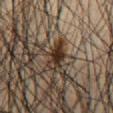<record>
<biopsy_status>not biopsied; imaged during a skin examination</biopsy_status>
<automated_metrics>
  <cielab_L>29</cielab_L>
  <cielab_a>14</cielab_a>
  <cielab_b>23</cielab_b>
  <vs_skin_darker_L>14.0</vs_skin_darker_L>
  <vs_skin_contrast_norm>13.0</vs_skin_contrast_norm>
  <lesion_detection_confidence_0_100>85</lesion_detection_confidence_0_100>
</automated_metrics>
<patient>
  <sex>male</sex>
  <age_approx>45</age_approx>
</patient>
<image>
  <source>total-body photography crop</source>
  <field_of_view_mm>15</field_of_view_mm>
</image>
<site>abdomen</site>
<lesion_size>
  <long_diameter_mm_approx>4.0</long_diameter_mm_approx>
</lesion_size>
<lighting>cross-polarized</lighting>
</record>